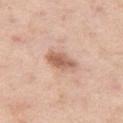Captured during whole-body skin photography for melanoma surveillance; the lesion was not biopsied.
An algorithmic analysis of the crop reported a footprint of about 7 mm², an eccentricity of roughly 0.85, and two-axis asymmetry of about 0.25. The software also gave an average lesion color of about L≈61 a*≈21 b*≈30 (CIELAB), a lesion–skin lightness drop of about 12, and a normalized border contrast of about 8.
A 15 mm close-up tile from a total-body photography series done for melanoma screening.
A female subject about 55 years old.
From the left thigh.
The lesion's longest dimension is about 4 mm.